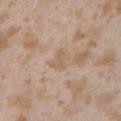From the arm.
A roughly 15 mm field-of-view crop from a total-body skin photograph.
The subject is a female in their mid-20s.
About 2.5 mm across.
An algorithmic analysis of the crop reported a mean CIELAB color near L≈60 a*≈15 b*≈30, a lesion–skin lightness drop of about 6, and a normalized lesion–skin contrast near 5. And it measured a border-irregularity rating of about 4/10, a color-variation rating of about 1.5/10, and radial color variation of about 0.5. The analysis additionally found a nevus-likeness score of about 0/100 and a lesion-detection confidence of about 100/100.
Captured under white-light illumination.